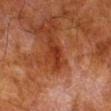biopsy status: no biopsy performed (imaged during a skin exam)
site: the right lower leg
illumination: cross-polarized
subject: male, in their 80s
image source: ~15 mm crop, total-body skin-cancer survey
lesion diameter: ~3.5 mm (longest diameter)